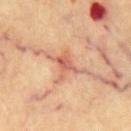Clinical impression:
Imaged during a routine full-body skin examination; the lesion was not biopsied and no histopathology is available.
Background:
The total-body-photography lesion software estimated a shape eccentricity near 0.7. Located on the front of the torso. The patient is a male aged 68–72. This is a cross-polarized tile. The lesion's longest dimension is about 5 mm. A close-up tile cropped from a whole-body skin photograph, about 15 mm across.